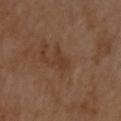Impression: Captured during whole-body skin photography for melanoma surveillance; the lesion was not biopsied. Background: The lesion is on the upper back. The lesion's longest dimension is about 2.5 mm. The lesion-visualizer software estimated a footprint of about 2.5 mm², an outline eccentricity of about 0.9 (0 = round, 1 = elongated), and a shape-asymmetry score of about 0.55 (0 = symmetric). And it measured a border-irregularity rating of about 5.5/10, a within-lesion color-variation index near 0/10, and radial color variation of about 0. It also reported a classifier nevus-likeness of about 0/100 and a detector confidence of about 100 out of 100 that the crop contains a lesion. This is a cross-polarized tile. A male patient aged around 55. A 15 mm close-up tile from a total-body photography series done for melanoma screening.